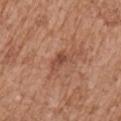notes = imaged on a skin check; not biopsied | acquisition = ~15 mm tile from a whole-body skin photo | lighting = white-light illumination | anatomic site = the right upper arm | lesion diameter = ≈3 mm | patient = female, roughly 75 years of age.